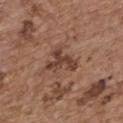workup=total-body-photography surveillance lesion; no biopsy | acquisition=total-body-photography crop, ~15 mm field of view | body site=the upper back | subject=female, approximately 65 years of age.